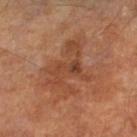Recorded during total-body skin imaging; not selected for excision or biopsy. The lesion is on the leg. A lesion tile, about 15 mm wide, cut from a 3D total-body photograph. A male patient, roughly 65 years of age.